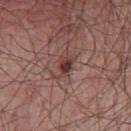{"image": {"source": "total-body photography crop", "field_of_view_mm": 15}, "lighting": "white-light", "automated_metrics": {"border_irregularity_0_10": 2.5, "color_variation_0_10": 4.5, "peripheral_color_asymmetry": 1.0, "nevus_likeness_0_100": 70, "lesion_detection_confidence_0_100": 100}, "patient": {"sex": "male", "age_approx": 65}, "site": "front of the torso", "lesion_size": {"long_diameter_mm_approx": 2.5}}